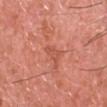Assessment:
Part of a total-body skin-imaging series; this lesion was reviewed on a skin check and was not flagged for biopsy.
Background:
From the head or neck. A male subject in their mid-40s. A 15 mm close-up tile from a total-body photography series done for melanoma screening.